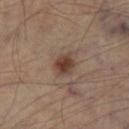{"patient": {"sex": "male", "age_approx": 55}, "site": "right thigh", "lighting": "cross-polarized", "lesion_size": {"long_diameter_mm_approx": 2.5}, "image": {"source": "total-body photography crop", "field_of_view_mm": 15}}